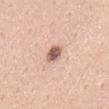Automated tile analysis of the lesion measured a footprint of about 4 mm², a shape eccentricity near 0.8, and a shape-asymmetry score of about 0.2 (0 = symmetric). Measured at roughly 2.5 mm in maximum diameter. A close-up tile cropped from a whole-body skin photograph, about 15 mm across. On the back. Captured under white-light illumination. A male patient, approximately 55 years of age.